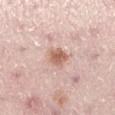Case summary:
* notes · catalogued during a skin exam; not biopsied
* site · the left lower leg
* subject · female, in their mid- to late 20s
* illumination · white-light illumination
* image-analysis metrics · border irregularity of about 2 on a 0–10 scale, internal color variation of about 2.5 on a 0–10 scale, and peripheral color asymmetry of about 1
* acquisition · total-body-photography crop, ~15 mm field of view